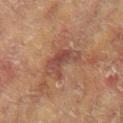<record>
<biopsy_status>not biopsied; imaged during a skin examination</biopsy_status>
<lighting>cross-polarized</lighting>
<site>arm</site>
<patient>
  <sex>female</sex>
  <age_approx>80</age_approx>
</patient>
<image>
  <source>total-body photography crop</source>
  <field_of_view_mm>15</field_of_view_mm>
</image>
</record>